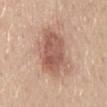Clinical impression:
Captured during whole-body skin photography for melanoma surveillance; the lesion was not biopsied.
Background:
A female subject, aged 43–47. Cropped from a total-body skin-imaging series; the visible field is about 15 mm. About 7 mm across. This is a white-light tile. Located on the mid back. Automated tile analysis of the lesion measured a mean CIELAB color near L≈58 a*≈21 b*≈28 and a lesion-to-skin contrast of about 8 (normalized; higher = more distinct). The software also gave border irregularity of about 3.5 on a 0–10 scale and internal color variation of about 5 on a 0–10 scale.